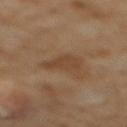Q: Is there a histopathology result?
A: catalogued during a skin exam; not biopsied
Q: Automated lesion metrics?
A: a lesion-to-skin contrast of about 6 (normalized; higher = more distinct); a border-irregularity rating of about 4/10 and a within-lesion color-variation index near 1/10; a detector confidence of about 100 out of 100 that the crop contains a lesion
Q: Lesion size?
A: about 3.5 mm
Q: Illumination type?
A: cross-polarized illumination
Q: Who is the patient?
A: female, aged 53 to 57
Q: What is the anatomic site?
A: the mid back
Q: What is the imaging modality?
A: total-body-photography crop, ~15 mm field of view